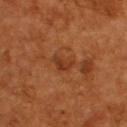This lesion was catalogued during total-body skin photography and was not selected for biopsy. Measured at roughly 3 mm in maximum diameter. A male subject, approximately 55 years of age. An algorithmic analysis of the crop reported an area of roughly 4 mm², an eccentricity of roughly 0.8, and a shape-asymmetry score of about 0.4 (0 = symmetric). The analysis additionally found an average lesion color of about L≈34 a*≈24 b*≈33 (CIELAB) and a lesion–skin lightness drop of about 7. The analysis additionally found a border-irregularity rating of about 3.5/10, a within-lesion color-variation index near 3/10, and radial color variation of about 1. The software also gave a classifier nevus-likeness of about 5/100 and lesion-presence confidence of about 100/100. Located on the upper back. Imaged with cross-polarized lighting. A region of skin cropped from a whole-body photographic capture, roughly 15 mm wide.